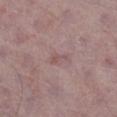<tbp_lesion>
  <biopsy_status>not biopsied; imaged during a skin examination</biopsy_status>
  <patient>
    <sex>male</sex>
    <age_approx>75</age_approx>
  </patient>
  <lighting>white-light</lighting>
  <lesion_size>
    <long_diameter_mm_approx>2.5</long_diameter_mm_approx>
  </lesion_size>
  <site>right lower leg</site>
  <image>
    <source>total-body photography crop</source>
    <field_of_view_mm>15</field_of_view_mm>
  </image>
</tbp_lesion>